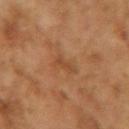– TBP lesion metrics · two-axis asymmetry of about 0.4; roughly 6 lightness units darker than nearby skin and a normalized lesion–skin contrast near 5.5; border irregularity of about 4.5 on a 0–10 scale, internal color variation of about 0 on a 0–10 scale, and peripheral color asymmetry of about 0; a nevus-likeness score of about 0/100 and a detector confidence of about 100 out of 100 that the crop contains a lesion
– location · the left upper arm
– patient · female, aged approximately 60
– image source · ~15 mm crop, total-body skin-cancer survey
– diameter · ≈3 mm